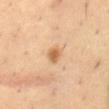Clinical impression: Captured during whole-body skin photography for melanoma surveillance; the lesion was not biopsied. Clinical summary: A male subject aged 48 to 52. The lesion is located on the abdomen. A region of skin cropped from a whole-body photographic capture, roughly 15 mm wide.